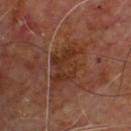Captured during whole-body skin photography for melanoma surveillance; the lesion was not biopsied. A 15 mm crop from a total-body photograph taken for skin-cancer surveillance. The lesion is on the upper back. A male subject approximately 60 years of age.